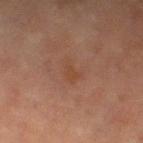Notes:
* biopsy status · imaged on a skin check; not biopsied
* automated metrics · a lesion area of about 4 mm² and an outline eccentricity of about 0.7 (0 = round, 1 = elongated); a border-irregularity index near 4/10 and peripheral color asymmetry of about 0.5
* diameter · about 3 mm
* image source · total-body-photography crop, ~15 mm field of view
* patient · female, approximately 70 years of age
* body site · the left thigh